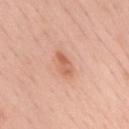subject = male, aged 53 to 57 | anatomic site = the mid back | image source = total-body-photography crop, ~15 mm field of view | diameter = ≈3.5 mm | automated metrics = a detector confidence of about 100 out of 100 that the crop contains a lesion.